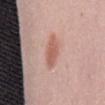Notes:
• notes · no biopsy performed (imaged during a skin exam)
• location · the mid back
• image source · 15 mm crop, total-body photography
• subject · female, aged 13–17